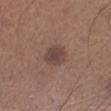| key | value |
|---|---|
| workup | no biopsy performed (imaged during a skin exam) |
| image source | total-body-photography crop, ~15 mm field of view |
| tile lighting | white-light |
| lesion size | about 3 mm |
| subject | male, approximately 65 years of age |
| site | the leg |